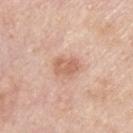Recorded during total-body skin imaging; not selected for excision or biopsy. A close-up tile cropped from a whole-body skin photograph, about 15 mm across. The tile uses white-light illumination. The lesion is located on the upper back. The patient is a male roughly 45 years of age. Measured at roughly 3 mm in maximum diameter.